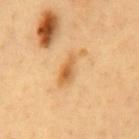  biopsy_status: not biopsied; imaged during a skin examination
  site: chest
  patient:
    sex: male
    age_approx: 60
  image:
    source: total-body photography crop
    field_of_view_mm: 15
  lighting: cross-polarized
  automated_metrics:
    cielab_L: 63
    cielab_a: 21
    cielab_b: 44
    vs_skin_darker_L: 10.0
  lesion_size:
    long_diameter_mm_approx: 5.0